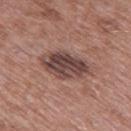Part of a total-body skin-imaging series; this lesion was reviewed on a skin check and was not flagged for biopsy.